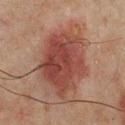Clinical impression: Imaged during a routine full-body skin examination; the lesion was not biopsied and no histopathology is available. Image and clinical context: Approximately 7.5 mm at its widest. A 15 mm crop from a total-body photograph taken for skin-cancer surveillance. The subject is a male aged around 65. The lesion is on the mid back. Captured under cross-polarized illumination.